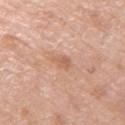Assessment:
Captured during whole-body skin photography for melanoma surveillance; the lesion was not biopsied.
Acquisition and patient details:
This is a white-light tile. The patient is a female aged approximately 75. Automated image analysis of the tile measured a mean CIELAB color near L≈61 a*≈22 b*≈32, roughly 8 lightness units darker than nearby skin, and a lesion-to-skin contrast of about 5.5 (normalized; higher = more distinct). The recorded lesion diameter is about 2.5 mm. Cropped from a whole-body photographic skin survey; the tile spans about 15 mm. The lesion is located on the right upper arm.